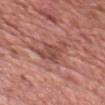A lesion tile, about 15 mm wide, cut from a 3D total-body photograph. The subject is a male about 50 years old. The lesion's longest dimension is about 4.5 mm. An algorithmic analysis of the crop reported a lesion area of about 8.5 mm² and two-axis asymmetry of about 0.4. The software also gave a lesion–skin lightness drop of about 9 and a lesion-to-skin contrast of about 7 (normalized; higher = more distinct). It also reported a classifier nevus-likeness of about 0/100 and a lesion-detection confidence of about 50/100. This is a white-light tile. Located on the head or neck.